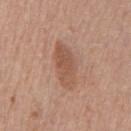follow-up: total-body-photography surveillance lesion; no biopsy | lesion diameter: about 5.5 mm | automated lesion analysis: an area of roughly 10 mm², an eccentricity of roughly 0.9, and a shape-asymmetry score of about 0.2 (0 = symmetric); a mean CIELAB color near L≈53 a*≈21 b*≈29, about 9 CIELAB-L* units darker than the surrounding skin, and a lesion-to-skin contrast of about 6.5 (normalized; higher = more distinct); an automated nevus-likeness rating near 40 out of 100 and a detector confidence of about 100 out of 100 that the crop contains a lesion | location: the chest | subject: male, aged approximately 65 | image: 15 mm crop, total-body photography.